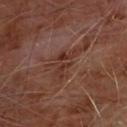biopsy status: no biopsy performed (imaged during a skin exam) | location: the chest | subject: male, about 60 years old | automated metrics: a lesion area of about 6 mm², a shape eccentricity near 0.85, and two-axis asymmetry of about 0.5; a lesion color around L≈32 a*≈21 b*≈24 in CIELAB and roughly 7 lightness units darker than nearby skin; internal color variation of about 3 on a 0–10 scale and radial color variation of about 1.5; an automated nevus-likeness rating near 0 out of 100 and a lesion-detection confidence of about 80/100 | image: ~15 mm crop, total-body skin-cancer survey | lighting: cross-polarized | diameter: ~4 mm (longest diameter).